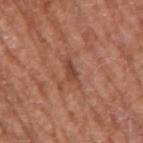- follow-up — no biopsy performed (imaged during a skin exam)
- lesion size — ~2.5 mm (longest diameter)
- automated metrics — an area of roughly 2.5 mm², an eccentricity of roughly 0.9, and two-axis asymmetry of about 0.35; a nevus-likeness score of about 0/100 and a lesion-detection confidence of about 95/100
- tile lighting — white-light illumination
- image — total-body-photography crop, ~15 mm field of view
- anatomic site — the right upper arm
- patient — male, in their mid- to late 60s